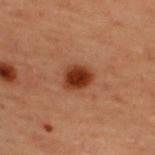biopsy status=imaged on a skin check; not biopsied.